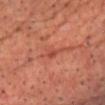| key | value |
|---|---|
| biopsy status | imaged on a skin check; not biopsied |
| site | the chest |
| image | ~15 mm crop, total-body skin-cancer survey |
| patient | male, aged around 65 |
| lighting | cross-polarized |